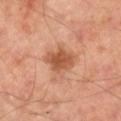This lesion was catalogued during total-body skin photography and was not selected for biopsy. A male patient, aged approximately 50. Measured at roughly 4.5 mm in maximum diameter. Automated image analysis of the tile measured a footprint of about 9 mm², an eccentricity of roughly 0.8, and a shape-asymmetry score of about 0.2 (0 = symmetric). And it measured an average lesion color of about L≈54 a*≈25 b*≈35 (CIELAB). And it measured border irregularity of about 2.5 on a 0–10 scale and internal color variation of about 3 on a 0–10 scale. It also reported a classifier nevus-likeness of about 20/100 and lesion-presence confidence of about 100/100. On the left leg. A 15 mm close-up extracted from a 3D total-body photography capture. The tile uses cross-polarized illumination.